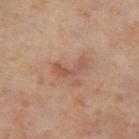Imaged during a routine full-body skin examination; the lesion was not biopsied and no histopathology is available.
A roughly 15 mm field-of-view crop from a total-body skin photograph.
Located on the right thigh.
A female patient about 55 years old.
The lesion's longest dimension is about 4 mm.